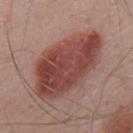notes: total-body-photography surveillance lesion; no biopsy
patient: male, in their mid- to late 50s
lesion size: about 10 mm
illumination: white-light illumination
image source: ~15 mm tile from a whole-body skin photo
body site: the mid back
automated lesion analysis: a border-irregularity rating of about 2/10, a within-lesion color-variation index near 5.5/10, and peripheral color asymmetry of about 2; a classifier nevus-likeness of about 100/100 and a detector confidence of about 100 out of 100 that the crop contains a lesion